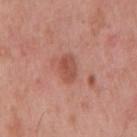• notes: total-body-photography surveillance lesion; no biopsy